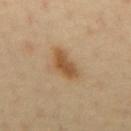Recorded during total-body skin imaging; not selected for excision or biopsy. A roughly 15 mm field-of-view crop from a total-body skin photograph. Captured under cross-polarized illumination. Approximately 4 mm at its widest. The lesion is located on the back. A male patient aged approximately 40.